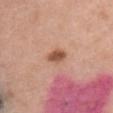<case>
  <biopsy_status>not biopsied; imaged during a skin examination</biopsy_status>
  <site>front of the torso</site>
  <image>
    <source>total-body photography crop</source>
    <field_of_view_mm>15</field_of_view_mm>
  </image>
  <patient>
    <sex>female</sex>
    <age_approx>35</age_approx>
  </patient>
</case>